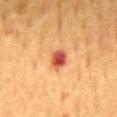Impression:
The lesion was photographed on a routine skin check and not biopsied; there is no pathology result.
Acquisition and patient details:
The patient is a male in their 60s. This is a cross-polarized tile. Automated image analysis of the tile measured a lesion area of about 4.5 mm², a shape eccentricity near 0.6, and a shape-asymmetry score of about 0.2 (0 = symmetric). And it measured a lesion color around L≈55 a*≈35 b*≈38 in CIELAB, roughly 17 lightness units darker than nearby skin, and a normalized lesion–skin contrast near 11. It also reported a within-lesion color-variation index near 8/10 and radial color variation of about 2.5. The software also gave an automated nevus-likeness rating near 0 out of 100 and a lesion-detection confidence of about 100/100. This image is a 15 mm lesion crop taken from a total-body photograph. Approximately 2.5 mm at its widest. The lesion is located on the mid back.